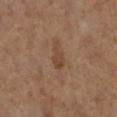biopsy_status: not biopsied; imaged during a skin examination
image:
  source: total-body photography crop
  field_of_view_mm: 15
patient:
  sex: female
  age_approx: 65
automated_metrics:
  area_mm2_approx: 4.0
  eccentricity: 0.85
  shape_asymmetry: 0.3
  cielab_L: 42
  cielab_a: 17
  cielab_b: 29
  vs_skin_darker_L: 7.0
  vs_skin_contrast_norm: 6.5
  nevus_likeness_0_100: 5
site: left lower leg
lighting: cross-polarized
lesion_size:
  long_diameter_mm_approx: 3.0